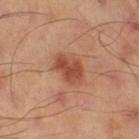Part of a total-body skin-imaging series; this lesion was reviewed on a skin check and was not flagged for biopsy.
On the right thigh.
Automated tile analysis of the lesion measured a lesion color around L≈50 a*≈27 b*≈34 in CIELAB, about 11 CIELAB-L* units darker than the surrounding skin, and a normalized border contrast of about 8. The software also gave a border-irregularity index near 2.5/10 and internal color variation of about 4 on a 0–10 scale.
The tile uses cross-polarized illumination.
Longest diameter approximately 4 mm.
Cropped from a whole-body photographic skin survey; the tile spans about 15 mm.